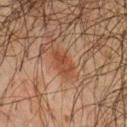The lesion was tiled from a total-body skin photograph and was not biopsied. About 3 mm across. A male subject, approximately 60 years of age. From the chest. The total-body-photography lesion software estimated a lesion color around L≈34 a*≈19 b*≈26 in CIELAB, a lesion–skin lightness drop of about 7, and a normalized border contrast of about 6.5. The analysis additionally found a border-irregularity index near 3/10, a color-variation rating of about 2.5/10, and peripheral color asymmetry of about 1. It also reported a nevus-likeness score of about 45/100 and a lesion-detection confidence of about 100/100. A region of skin cropped from a whole-body photographic capture, roughly 15 mm wide.